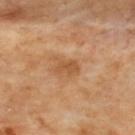Case summary:
– notes: total-body-photography surveillance lesion; no biopsy